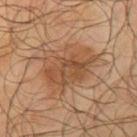Q: Was this lesion biopsied?
A: total-body-photography surveillance lesion; no biopsy
Q: What kind of image is this?
A: total-body-photography crop, ~15 mm field of view
Q: What lighting was used for the tile?
A: cross-polarized illumination
Q: What are the patient's age and sex?
A: male, aged 63–67
Q: Lesion size?
A: about 6.5 mm
Q: Lesion location?
A: the arm